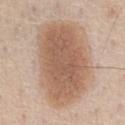Assessment:
Captured during whole-body skin photography for melanoma surveillance; the lesion was not biopsied.